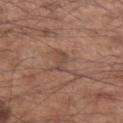Assessment: Imaged during a routine full-body skin examination; the lesion was not biopsied and no histopathology is available. Acquisition and patient details: The lesion-visualizer software estimated an area of roughly 3.5 mm², an eccentricity of roughly 0.95, and a symmetry-axis asymmetry near 0.3. And it measured border irregularity of about 4 on a 0–10 scale, a within-lesion color-variation index near 0.5/10, and a peripheral color-asymmetry measure near 0. The software also gave a classifier nevus-likeness of about 0/100 and a detector confidence of about 95 out of 100 that the crop contains a lesion. This image is a 15 mm lesion crop taken from a total-body photograph. Measured at roughly 3.5 mm in maximum diameter. The lesion is located on the left forearm. A male subject aged approximately 55.